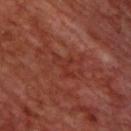Notes:
* biopsy status — total-body-photography surveillance lesion; no biopsy
* size — about 3 mm
* lighting — cross-polarized
* imaging modality — ~15 mm crop, total-body skin-cancer survey
* patient — male, in their 70s
* anatomic site — the upper back